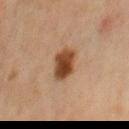{
  "biopsy_status": "not biopsied; imaged during a skin examination",
  "automated_metrics": {
    "area_mm2_approx": 8.0,
    "eccentricity": 0.55,
    "shape_asymmetry": 0.25,
    "cielab_L": 36,
    "cielab_a": 18,
    "cielab_b": 29,
    "vs_skin_darker_L": 14.0,
    "nevus_likeness_0_100": 100,
    "lesion_detection_confidence_0_100": 100
  },
  "patient": {
    "sex": "female",
    "age_approx": 40
  },
  "image": {
    "source": "total-body photography crop",
    "field_of_view_mm": 15
  },
  "lesion_size": {
    "long_diameter_mm_approx": 3.5
  },
  "lighting": "cross-polarized",
  "site": "front of the torso"
}